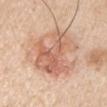No biopsy was performed on this lesion — it was imaged during a full skin examination and was not determined to be concerning.
The tile uses white-light illumination.
About 7.5 mm across.
The lesion-visualizer software estimated an area of roughly 35 mm² and an eccentricity of roughly 0.55. The analysis additionally found an average lesion color of about L≈65 a*≈21 b*≈31 (CIELAB), a lesion–skin lightness drop of about 10, and a lesion-to-skin contrast of about 6.5 (normalized; higher = more distinct). It also reported a border-irregularity rating of about 4/10, internal color variation of about 7.5 on a 0–10 scale, and a peripheral color-asymmetry measure near 2.5. It also reported a nevus-likeness score of about 5/100 and a lesion-detection confidence of about 100/100.
A male subject, about 60 years old.
From the right upper arm.
This image is a 15 mm lesion crop taken from a total-body photograph.